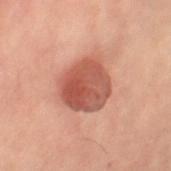No biopsy was performed on this lesion — it was imaged during a full skin examination and was not determined to be concerning. Automated image analysis of the tile measured a footprint of about 19 mm², an outline eccentricity of about 0.45 (0 = round, 1 = elongated), and a symmetry-axis asymmetry near 0.15. The analysis additionally found an automated nevus-likeness rating near 95 out of 100 and a lesion-detection confidence of about 100/100. A 15 mm crop from a total-body photograph taken for skin-cancer surveillance. Captured under cross-polarized illumination. The lesion is located on the left leg. The subject is a female aged 58 to 62. The recorded lesion diameter is about 5 mm.